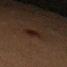The lesion was photographed on a routine skin check and not biopsied; there is no pathology result.
Imaged with cross-polarized lighting.
Cropped from a whole-body photographic skin survey; the tile spans about 15 mm.
The lesion is located on the left thigh.
The recorded lesion diameter is about 3 mm.
A female subject, aged approximately 40.